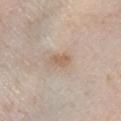No biopsy was performed on this lesion — it was imaged during a full skin examination and was not determined to be concerning.
Automated tile analysis of the lesion measured a lesion area of about 4 mm² and a symmetry-axis asymmetry near 0.25. And it measured a mean CIELAB color near L≈61 a*≈15 b*≈29 and a lesion-to-skin contrast of about 6.5 (normalized; higher = more distinct). The software also gave a border-irregularity index near 2.5/10, internal color variation of about 1.5 on a 0–10 scale, and peripheral color asymmetry of about 0.5.
Located on the right lower leg.
A female patient, aged approximately 55.
This is a white-light tile.
A region of skin cropped from a whole-body photographic capture, roughly 15 mm wide.